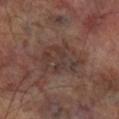notes: no biopsy performed (imaged during a skin exam)
location: the right lower leg
image source: 15 mm crop, total-body photography
subject: male, aged approximately 70